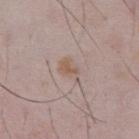  biopsy_status: not biopsied; imaged during a skin examination
  patient:
    sex: male
    age_approx: 50
  image:
    source: total-body photography crop
    field_of_view_mm: 15
  lesion_size:
    long_diameter_mm_approx: 3.0
  lighting: white-light
  site: chest
  automated_metrics:
    eccentricity: 0.75
    shape_asymmetry: 0.35
    cielab_L: 56
    cielab_a: 15
    cielab_b: 25
    vs_skin_darker_L: 7.0
    vs_skin_contrast_norm: 6.5
    border_irregularity_0_10: 3.5
    color_variation_0_10: 2.5
    peripheral_color_asymmetry: 1.0